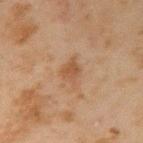{
  "biopsy_status": "not biopsied; imaged during a skin examination",
  "patient": {
    "sex": "male",
    "age_approx": 45
  },
  "image": {
    "source": "total-body photography crop",
    "field_of_view_mm": 15
  },
  "site": "right upper arm",
  "automated_metrics": {
    "area_mm2_approx": 5.5,
    "eccentricity": 0.7,
    "shape_asymmetry": 0.35,
    "cielab_L": 43,
    "cielab_a": 17,
    "cielab_b": 29,
    "vs_skin_darker_L": 6.0,
    "vs_skin_contrast_norm": 5.5,
    "nevus_likeness_0_100": 5,
    "lesion_detection_confidence_0_100": 100
  },
  "lesion_size": {
    "long_diameter_mm_approx": 3.5
  }
}